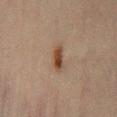workup — imaged on a skin check; not biopsied | diameter — about 3.5 mm | patient — male, aged approximately 45 | automated lesion analysis — an average lesion color of about L≈36 a*≈16 b*≈25 (CIELAB), a lesion–skin lightness drop of about 10, and a normalized lesion–skin contrast near 9; a border-irregularity index near 3.5/10 and radial color variation of about 1; an automated nevus-likeness rating near 95 out of 100 and lesion-presence confidence of about 100/100 | location — the abdomen | illumination — cross-polarized | image — 15 mm crop, total-body photography.